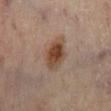A roughly 15 mm field-of-view crop from a total-body skin photograph.
A female subject aged approximately 60.
From the right lower leg.
About 4 mm across.
The total-body-photography lesion software estimated a lesion color around L≈44 a*≈18 b*≈29 in CIELAB. And it measured a within-lesion color-variation index near 6/10 and a peripheral color-asymmetry measure near 2. The analysis additionally found an automated nevus-likeness rating near 95 out of 100.
Captured under cross-polarized illumination.